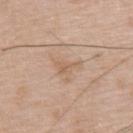Impression: The lesion was photographed on a routine skin check and not biopsied; there is no pathology result. Background: This is a white-light tile. A roughly 15 mm field-of-view crop from a total-body skin photograph. The lesion-visualizer software estimated an eccentricity of roughly 0.85 and a shape-asymmetry score of about 0.6 (0 = symmetric). And it measured a border-irregularity rating of about 6.5/10 and internal color variation of about 2 on a 0–10 scale. The software also gave a nevus-likeness score of about 0/100 and a detector confidence of about 100 out of 100 that the crop contains a lesion. The subject is a male aged approximately 75. The lesion is on the upper back. Longest diameter approximately 3 mm.